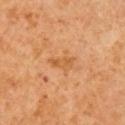Clinical summary: Imaged with cross-polarized lighting. Automated image analysis of the tile measured a mean CIELAB color near L≈56 a*≈24 b*≈43, a lesion–skin lightness drop of about 7, and a normalized lesion–skin contrast near 6. The analysis additionally found a border-irregularity index near 4/10, a within-lesion color-variation index near 1/10, and radial color variation of about 0.5. It also reported a nevus-likeness score of about 0/100 and a detector confidence of about 100 out of 100 that the crop contains a lesion. A 15 mm crop from a total-body photograph taken for skin-cancer surveillance. The subject is a male approximately 60 years of age. From the right upper arm. Approximately 3 mm at its widest.